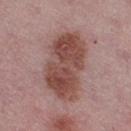Notes:
– follow-up · imaged on a skin check; not biopsied
– body site · the right thigh
– TBP lesion metrics · about 12 CIELAB-L* units darker than the surrounding skin and a normalized lesion–skin contrast near 9.5
– subject · female, roughly 45 years of age
– image · ~15 mm crop, total-body skin-cancer survey
– lesion size · ~8.5 mm (longest diameter)
– tile lighting · white-light